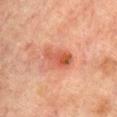Case summary:
* image: total-body-photography crop, ~15 mm field of view
* lighting: cross-polarized
* automated lesion analysis: an outline eccentricity of about 0.85 (0 = round, 1 = elongated) and a symmetry-axis asymmetry near 0.3; a classifier nevus-likeness of about 35/100 and a detector confidence of about 100 out of 100 that the crop contains a lesion
* patient: male, approximately 65 years of age
* location: the back
* lesion diameter: ≈4 mm